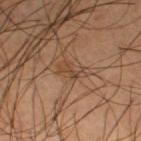Q: Was a biopsy performed?
A: total-body-photography surveillance lesion; no biopsy
Q: Where on the body is the lesion?
A: the left thigh
Q: Who is the patient?
A: male, in their mid- to late 50s
Q: What kind of image is this?
A: ~15 mm tile from a whole-body skin photo